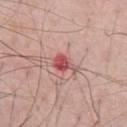{"biopsy_status": "not biopsied; imaged during a skin examination"}